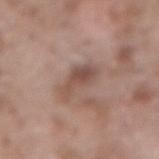{
  "biopsy_status": "not biopsied; imaged during a skin examination",
  "lighting": "white-light",
  "image": {
    "source": "total-body photography crop",
    "field_of_view_mm": 15
  },
  "lesion_size": {
    "long_diameter_mm_approx": 5.0
  },
  "site": "lower back",
  "patient": {
    "sex": "male",
    "age_approx": 55
  }
}